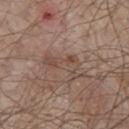biopsy status: imaged on a skin check; not biopsied
subject: male, aged approximately 80
diameter: about 5.5 mm
site: the chest
image source: ~15 mm tile from a whole-body skin photo
tile lighting: white-light illumination